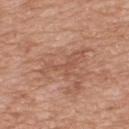  biopsy_status: not biopsied; imaged during a skin examination
  image:
    source: total-body photography crop
    field_of_view_mm: 15
  patient:
    sex: male
    age_approx: 50
  site: upper back
  lesion_size:
    long_diameter_mm_approx: 5.5
  lighting: white-light